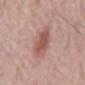The lesion was tiled from a total-body skin photograph and was not biopsied.
Approximately 4 mm at its widest.
This is a white-light tile.
The lesion is on the mid back.
The patient is a male aged 63 to 67.
An algorithmic analysis of the crop reported a border-irregularity rating of about 2/10, internal color variation of about 2.5 on a 0–10 scale, and radial color variation of about 0.5. It also reported a nevus-likeness score of about 90/100 and a detector confidence of about 100 out of 100 that the crop contains a lesion.
A lesion tile, about 15 mm wide, cut from a 3D total-body photograph.